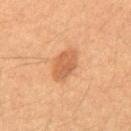| field | value |
|---|---|
| notes | total-body-photography surveillance lesion; no biopsy |
| TBP lesion metrics | an area of roughly 7 mm², an eccentricity of roughly 0.65, and a symmetry-axis asymmetry near 0.2 |
| location | the abdomen |
| image source | 15 mm crop, total-body photography |
| lesion diameter | ≈3.5 mm |
| patient | male, roughly 50 years of age |